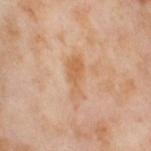| feature | finding |
|---|---|
| follow-up | catalogued during a skin exam; not biopsied |
| tile lighting | cross-polarized illumination |
| acquisition | total-body-photography crop, ~15 mm field of view |
| site | the right thigh |
| subject | female, in their mid-50s |